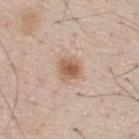Q: Was a biopsy performed?
A: imaged on a skin check; not biopsied
Q: How was this image acquired?
A: ~15 mm tile from a whole-body skin photo
Q: How large is the lesion?
A: about 2.5 mm
Q: What lighting was used for the tile?
A: white-light
Q: What is the anatomic site?
A: the upper back
Q: What are the patient's age and sex?
A: male, aged 73–77